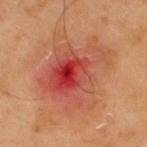| key | value |
|---|---|
| biopsy status | catalogued during a skin exam; not biopsied |
| acquisition | 15 mm crop, total-body photography |
| lesion diameter | ~9 mm (longest diameter) |
| location | the upper back |
| subject | male, about 40 years old |
| automated lesion analysis | a shape eccentricity near 0.6 and a symmetry-axis asymmetry near 0.25; a border-irregularity index near 4.5/10, a within-lesion color-variation index near 10/10, and a peripheral color-asymmetry measure near 4.5 |
| lighting | cross-polarized |